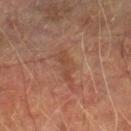Findings:
- follow-up: no biopsy performed (imaged during a skin exam)
- imaging modality: total-body-photography crop, ~15 mm field of view
- size: ~4 mm (longest diameter)
- subject: male, roughly 75 years of age
- illumination: cross-polarized
- automated metrics: a normalized lesion–skin contrast near 5; a border-irregularity index near 4.5/10 and a peripheral color-asymmetry measure near 0.5; a lesion-detection confidence of about 100/100
- anatomic site: the right lower leg